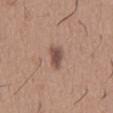This lesion was catalogued during total-body skin photography and was not selected for biopsy.
A male patient, roughly 65 years of age.
A 15 mm close-up tile from a total-body photography series done for melanoma screening.
Imaged with white-light lighting.
Located on the mid back.